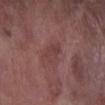This lesion was catalogued during total-body skin photography and was not selected for biopsy. A male subject in their mid- to late 70s. This is a white-light tile. The lesion is on the left lower leg. Approximately 3 mm at its widest. Cropped from a whole-body photographic skin survey; the tile spans about 15 mm.